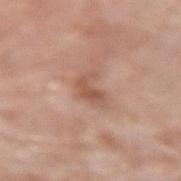workup = catalogued during a skin exam; not biopsied | subject = female, approximately 75 years of age | imaging modality = 15 mm crop, total-body photography | anatomic site = the left forearm | size = ~3 mm (longest diameter) | image-analysis metrics = a border-irregularity index near 5.5/10 and a within-lesion color-variation index near 2.5/10; a classifier nevus-likeness of about 0/100 and a lesion-detection confidence of about 100/100.